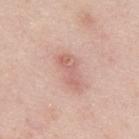The lesion was tiled from a total-body skin photograph and was not biopsied. A close-up tile cropped from a whole-body skin photograph, about 15 mm across. From the upper back. The subject is a male aged around 25.